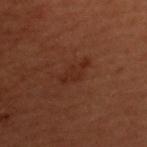Part of a total-body skin-imaging series; this lesion was reviewed on a skin check and was not flagged for biopsy.
This image is a 15 mm lesion crop taken from a total-body photograph.
The lesion is located on the upper back.
A female patient, aged 48–52.